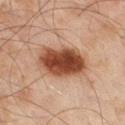The lesion was tiled from a total-body skin photograph and was not biopsied.
Automated image analysis of the tile measured a footprint of about 18 mm², an eccentricity of roughly 0.7, and a symmetry-axis asymmetry near 0.15. And it measured an average lesion color of about L≈42 a*≈23 b*≈30 (CIELAB), about 17 CIELAB-L* units darker than the surrounding skin, and a normalized lesion–skin contrast near 13.
The tile uses cross-polarized illumination.
The lesion is located on the right thigh.
Cropped from a whole-body photographic skin survey; the tile spans about 15 mm.
A male subject, roughly 60 years of age.